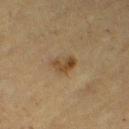anatomic site — the right thigh | image — ~15 mm tile from a whole-body skin photo | subject — female, approximately 55 years of age.